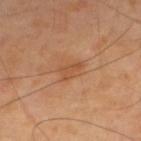Impression:
Recorded during total-body skin imaging; not selected for excision or biopsy.
Background:
The tile uses cross-polarized illumination. A male patient, aged 38–42. About 3 mm across. A 15 mm close-up tile from a total-body photography series done for melanoma screening. The lesion is on the upper back.